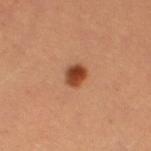Clinical impression: This lesion was catalogued during total-body skin photography and was not selected for biopsy. Clinical summary: Automated tile analysis of the lesion measured a lesion area of about 4.5 mm², an eccentricity of roughly 0.45, and two-axis asymmetry of about 0.2. It also reported a border-irregularity index near 1.5/10, a within-lesion color-variation index near 4/10, and radial color variation of about 1. The analysis additionally found a detector confidence of about 100 out of 100 that the crop contains a lesion. From the left thigh. A 15 mm close-up tile from a total-body photography series done for melanoma screening. The lesion's longest dimension is about 2.5 mm. A female subject, in their mid-20s.